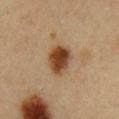No biopsy was performed on this lesion — it was imaged during a full skin examination and was not determined to be concerning. Cropped from a total-body skin-imaging series; the visible field is about 15 mm. Approximately 4 mm at its widest. From the chest. A male patient, roughly 50 years of age. This is a cross-polarized tile.